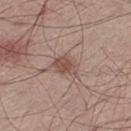- notes · total-body-photography surveillance lesion; no biopsy
- image-analysis metrics · internal color variation of about 2 on a 0–10 scale and a peripheral color-asymmetry measure near 0.5; an automated nevus-likeness rating near 80 out of 100 and lesion-presence confidence of about 100/100
- tile lighting · white-light illumination
- site · the left thigh
- image · total-body-photography crop, ~15 mm field of view
- subject · male, approximately 55 years of age